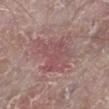{
  "lighting": "white-light",
  "site": "left lower leg",
  "automated_metrics": {
    "cielab_L": 50,
    "cielab_a": 23,
    "cielab_b": 18,
    "vs_skin_darker_L": 7.0
  },
  "lesion_size": {
    "long_diameter_mm_approx": 5.0
  },
  "patient": {
    "sex": "male",
    "age_approx": 65
  },
  "image": {
    "source": "total-body photography crop",
    "field_of_view_mm": 15
  }
}